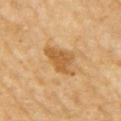Captured during whole-body skin photography for melanoma surveillance; the lesion was not biopsied.
The patient is a female about 70 years old.
From the right upper arm.
A 15 mm close-up tile from a total-body photography series done for melanoma screening.
Measured at roughly 4 mm in maximum diameter.
This is a cross-polarized tile.
Automated tile analysis of the lesion measured a mean CIELAB color near L≈60 a*≈21 b*≈45, a lesion–skin lightness drop of about 11, and a normalized lesion–skin contrast near 7.5.